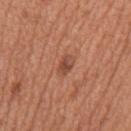biopsy status — imaged on a skin check; not biopsied | site — the mid back | acquisition — 15 mm crop, total-body photography | illumination — white-light | subject — male, roughly 65 years of age.